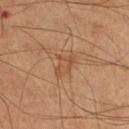Case summary:
* workup: imaged on a skin check; not biopsied
* body site: the left lower leg
* illumination: cross-polarized
* subject: male, approximately 60 years of age
* acquisition: ~15 mm tile from a whole-body skin photo
* size: ~3.5 mm (longest diameter)